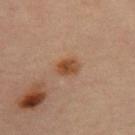Clinical impression:
This lesion was catalogued during total-body skin photography and was not selected for biopsy.